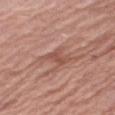This lesion was catalogued during total-body skin photography and was not selected for biopsy. The subject is a male approximately 80 years of age. Approximately 3.5 mm at its widest. On the right thigh. A 15 mm crop from a total-body photograph taken for skin-cancer surveillance. This is a white-light tile.